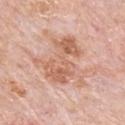subject: male, aged 78 to 82 | image source: ~15 mm crop, total-body skin-cancer survey | automated lesion analysis: an outline eccentricity of about 0.2 (0 = round, 1 = elongated) and two-axis asymmetry of about 0.55; an average lesion color of about L≈63 a*≈22 b*≈31 (CIELAB) and a normalized border contrast of about 6.5; lesion-presence confidence of about 100/100 | lighting: white-light illumination | lesion size: ≈7 mm | body site: the mid back.